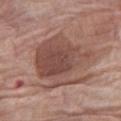biopsy status: total-body-photography surveillance lesion; no biopsy | image source: 15 mm crop, total-body photography | body site: the right thigh | lesion size: about 7.5 mm | illumination: white-light | TBP lesion metrics: a lesion area of about 30 mm², an outline eccentricity of about 0.55 (0 = round, 1 = elongated), and a shape-asymmetry score of about 0.35 (0 = symmetric); a lesion color around L≈47 a*≈20 b*≈24 in CIELAB and a lesion–skin lightness drop of about 10 | subject: female, aged 73 to 77.